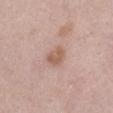The lesion was photographed on a routine skin check and not biopsied; there is no pathology result.
The patient is a female about 65 years old.
The lesion's longest dimension is about 3 mm.
From the front of the torso.
The tile uses white-light illumination.
A close-up tile cropped from a whole-body skin photograph, about 15 mm across.